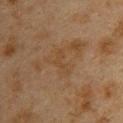Impression:
Captured during whole-body skin photography for melanoma surveillance; the lesion was not biopsied.
Image and clinical context:
The lesion-visualizer software estimated an area of roughly 3.5 mm², a shape eccentricity near 0.9, and a shape-asymmetry score of about 0.6 (0 = symmetric). The analysis additionally found a nevus-likeness score of about 0/100. Located on the upper back. A female patient about 40 years old. The tile uses cross-polarized illumination. A lesion tile, about 15 mm wide, cut from a 3D total-body photograph. Measured at roughly 3.5 mm in maximum diameter.